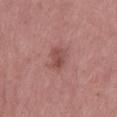workup: no biopsy performed (imaged during a skin exam)
subject: female, roughly 65 years of age
site: the left thigh
tile lighting: white-light
TBP lesion metrics: a shape eccentricity near 0.85 and a symmetry-axis asymmetry near 0.2; a lesion color around L≈49 a*≈24 b*≈24 in CIELAB, a lesion–skin lightness drop of about 9, and a lesion-to-skin contrast of about 6.5 (normalized; higher = more distinct); a border-irregularity rating of about 2.5/10, internal color variation of about 3.5 on a 0–10 scale, and radial color variation of about 1.5
image source: total-body-photography crop, ~15 mm field of view